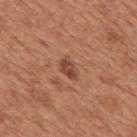  biopsy_status: not biopsied; imaged during a skin examination
  lighting: white-light
  automated_metrics:
    vs_skin_darker_L: 10.0
    vs_skin_contrast_norm: 7.5
    border_irregularity_0_10: 2.5
    color_variation_0_10: 2.0
    nevus_likeness_0_100: 50
  lesion_size:
    long_diameter_mm_approx: 3.0
  patient:
    sex: male
    age_approx: 65
  image:
    source: total-body photography crop
    field_of_view_mm: 15
  site: mid back